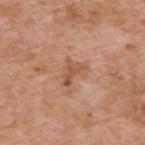Impression: The lesion was tiled from a total-body skin photograph and was not biopsied. Background: Imaged with white-light lighting. From the upper back. A lesion tile, about 15 mm wide, cut from a 3D total-body photograph. The total-body-photography lesion software estimated a footprint of about 5 mm², a shape eccentricity near 0.75, and a symmetry-axis asymmetry near 0.55. It also reported an average lesion color of about L≈54 a*≈22 b*≈33 (CIELAB), roughly 8 lightness units darker than nearby skin, and a normalized lesion–skin contrast near 6. The software also gave a border-irregularity rating of about 5.5/10 and radial color variation of about 0.5. The analysis additionally found a nevus-likeness score of about 0/100 and a lesion-detection confidence of about 100/100. A male subject, in their 60s. About 3.5 mm across.